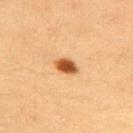notes: imaged on a skin check; not biopsied
image source: total-body-photography crop, ~15 mm field of view
lighting: cross-polarized
subject: female, approximately 35 years of age
size: about 3 mm
location: the right upper arm